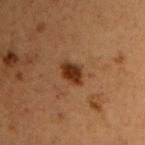Assessment:
This lesion was catalogued during total-body skin photography and was not selected for biopsy.